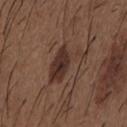The lesion was photographed on a routine skin check and not biopsied; there is no pathology result.
Cropped from a whole-body photographic skin survey; the tile spans about 15 mm.
From the front of the torso.
A male patient, roughly 50 years of age.